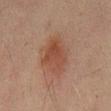No biopsy was performed on this lesion — it was imaged during a full skin examination and was not determined to be concerning. On the back. Imaged with cross-polarized lighting. The lesion's longest dimension is about 6 mm. A male patient roughly 45 years of age. A 15 mm crop from a total-body photograph taken for skin-cancer surveillance.